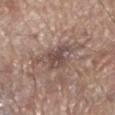Findings:
- follow-up · imaged on a skin check; not biopsied
- TBP lesion metrics · a footprint of about 11 mm², a shape eccentricity near 0.65, and two-axis asymmetry of about 0.45
- tile lighting · white-light illumination
- subject · male, aged 68 to 72
- location · the left lower leg
- size · ~5 mm (longest diameter)
- acquisition · ~15 mm tile from a whole-body skin photo